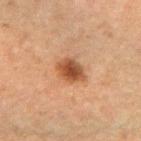{
  "biopsy_status": "not biopsied; imaged during a skin examination",
  "lesion_size": {
    "long_diameter_mm_approx": 3.5
  },
  "lighting": "cross-polarized",
  "image": {
    "source": "total-body photography crop",
    "field_of_view_mm": 15
  },
  "patient": {
    "sex": "female",
    "age_approx": 40
  },
  "site": "right forearm",
  "automated_metrics": {
    "cielab_L": 42,
    "cielab_a": 21,
    "cielab_b": 31,
    "vs_skin_darker_L": 12.0,
    "vs_skin_contrast_norm": 10.0,
    "nevus_likeness_0_100": 95
  }
}